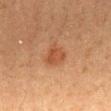biopsy status: catalogued during a skin exam; not biopsied
image: total-body-photography crop, ~15 mm field of view
size: ≈3 mm
body site: the mid back
patient: male, about 35 years old
lighting: cross-polarized illumination